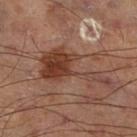  biopsy_status: not biopsied; imaged during a skin examination
  lighting: cross-polarized
  lesion_size:
    long_diameter_mm_approx: 9.0
  image:
    source: total-body photography crop
    field_of_view_mm: 15
  site: right thigh
  automated_metrics:
    area_mm2_approx: 18.0
    eccentricity: 0.9
    shape_asymmetry: 0.7
    cielab_L: 41
    cielab_a: 22
    cielab_b: 28